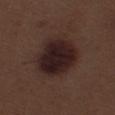Clinical impression:
Part of a total-body skin-imaging series; this lesion was reviewed on a skin check and was not flagged for biopsy.
Clinical summary:
The total-body-photography lesion software estimated an outline eccentricity of about 0.5 (0 = round, 1 = elongated) and a symmetry-axis asymmetry near 0.15. The analysis additionally found a mean CIELAB color near L≈21 a*≈15 b*≈15, roughly 11 lightness units darker than nearby skin, and a lesion-to-skin contrast of about 13.5 (normalized; higher = more distinct). It also reported a border-irregularity rating of about 1.5/10, a color-variation rating of about 5/10, and a peripheral color-asymmetry measure near 1.5. The analysis additionally found a nevus-likeness score of about 85/100. A male subject roughly 70 years of age. About 5.5 mm across. This image is a 15 mm lesion crop taken from a total-body photograph. From the left thigh.